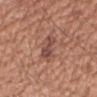<tbp_lesion>
<biopsy_status>not biopsied; imaged during a skin examination</biopsy_status>
<lighting>white-light</lighting>
<site>right upper arm</site>
<image>
  <source>total-body photography crop</source>
  <field_of_view_mm>15</field_of_view_mm>
</image>
<patient>
  <sex>male</sex>
  <age_approx>50</age_approx>
</patient>
<automated_metrics>
  <area_mm2_approx>6.5</area_mm2_approx>
  <eccentricity>0.9</eccentricity>
  <shape_asymmetry>0.4</shape_asymmetry>
</automated_metrics>
<lesion_size>
  <long_diameter_mm_approx>4.0</long_diameter_mm_approx>
</lesion_size>
</tbp_lesion>